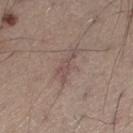Imaged during a routine full-body skin examination; the lesion was not biopsied and no histopathology is available.